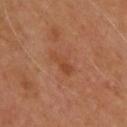The lesion was photographed on a routine skin check and not biopsied; there is no pathology result. Imaged with cross-polarized lighting. Located on the upper back. A lesion tile, about 15 mm wide, cut from a 3D total-body photograph. A male subject, aged 68 to 72. The lesion's longest dimension is about 3.5 mm.